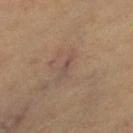Q: Was this lesion biopsied?
A: imaged on a skin check; not biopsied
Q: Lesion location?
A: the leg
Q: What is the imaging modality?
A: ~15 mm tile from a whole-body skin photo
Q: Patient demographics?
A: female, aged approximately 50